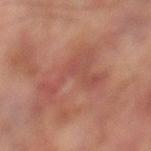Part of a total-body skin-imaging series; this lesion was reviewed on a skin check and was not flagged for biopsy.
Approximately 7 mm at its widest.
A 15 mm crop from a total-body photograph taken for skin-cancer surveillance.
Located on the left lower leg.
The subject is a male approximately 75 years of age.